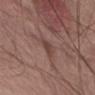The lesion was tiled from a total-body skin photograph and was not biopsied. About 2.5 mm across. The patient is a male aged 68 to 72. Cropped from a whole-body photographic skin survey; the tile spans about 15 mm. Located on the chest.